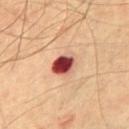This lesion was catalogued during total-body skin photography and was not selected for biopsy.
The lesion is located on the chest.
A male subject, aged 63–67.
Imaged with cross-polarized lighting.
Longest diameter approximately 3 mm.
A region of skin cropped from a whole-body photographic capture, roughly 15 mm wide.